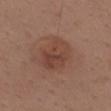biopsy status = imaged on a skin check; not biopsied
body site = the back
patient = male, roughly 40 years of age
image = total-body-photography crop, ~15 mm field of view